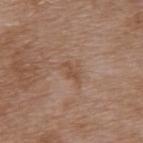Part of a total-body skin-imaging series; this lesion was reviewed on a skin check and was not flagged for biopsy. Located on the back. A lesion tile, about 15 mm wide, cut from a 3D total-body photograph. A female subject aged 68 to 72.